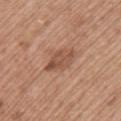This lesion was catalogued during total-body skin photography and was not selected for biopsy.
A 15 mm close-up tile from a total-body photography series done for melanoma screening.
The lesion is located on the upper back.
The total-body-photography lesion software estimated a nevus-likeness score of about 10/100 and a lesion-detection confidence of about 100/100.
A female patient aged 58–62.
The lesion's longest dimension is about 4.5 mm.
Imaged with white-light lighting.